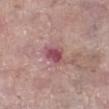patient:
  sex: female
  age_approx: 60
lesion_size:
  long_diameter_mm_approx: 3.5
site: leg
image:
  source: total-body photography crop
  field_of_view_mm: 15